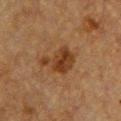{"biopsy_status": "not biopsied; imaged during a skin examination", "site": "chest", "image": {"source": "total-body photography crop", "field_of_view_mm": 15}, "automated_metrics": {"cielab_L": 31, "cielab_a": 18, "cielab_b": 29, "vs_skin_darker_L": 9.0, "vs_skin_contrast_norm": 8.5, "border_irregularity_0_10": 4.5, "color_variation_0_10": 4.5, "peripheral_color_asymmetry": 1.5, "nevus_likeness_0_100": 80, "lesion_detection_confidence_0_100": 100}, "lesion_size": {"long_diameter_mm_approx": 4.0}, "patient": {"sex": "female", "age_approx": 55}, "lighting": "cross-polarized"}